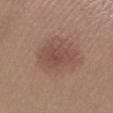Recorded during total-body skin imaging; not selected for excision or biopsy.
Imaged with white-light lighting.
A female patient, roughly 40 years of age.
Located on the right lower leg.
The lesion's longest dimension is about 4.5 mm.
A lesion tile, about 15 mm wide, cut from a 3D total-body photograph.
Automated tile analysis of the lesion measured a within-lesion color-variation index near 2.5/10 and a peripheral color-asymmetry measure near 1. And it measured a lesion-detection confidence of about 100/100.